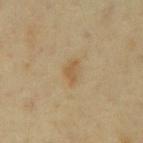{"biopsy_status": "not biopsied; imaged during a skin examination", "site": "chest", "image": {"source": "total-body photography crop", "field_of_view_mm": 15}, "patient": {"sex": "male", "age_approx": 65}, "lesion_size": {"long_diameter_mm_approx": 2.5}}